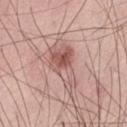Q: What is the imaging modality?
A: total-body-photography crop, ~15 mm field of view
Q: Automated lesion metrics?
A: an area of roughly 14 mm² and a shape-asymmetry score of about 0.35 (0 = symmetric); a border-irregularity rating of about 4/10 and a within-lesion color-variation index near 9/10
Q: What is the lesion's diameter?
A: ≈6.5 mm
Q: What lighting was used for the tile?
A: white-light illumination
Q: Where on the body is the lesion?
A: the leg
Q: What are the patient's age and sex?
A: male, aged 58–62